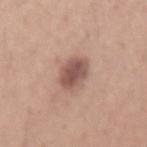The lesion was tiled from a total-body skin photograph and was not biopsied.
A region of skin cropped from a whole-body photographic capture, roughly 15 mm wide.
From the lower back.
A male patient, aged around 30.